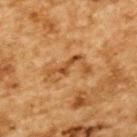Captured during whole-body skin photography for melanoma surveillance; the lesion was not biopsied.
Automated image analysis of the tile measured a lesion area of about 8.5 mm² and a shape-asymmetry score of about 0.5 (0 = symmetric).
A male subject about 85 years old or older.
A 15 mm crop from a total-body photograph taken for skin-cancer surveillance.
The lesion is on the upper back.
Captured under cross-polarized illumination.
Approximately 5.5 mm at its widest.